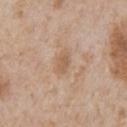| key | value |
|---|---|
| biopsy status | total-body-photography surveillance lesion; no biopsy |
| subject | male, in their mid-60s |
| diameter | ≈2.5 mm |
| site | the front of the torso |
| acquisition | total-body-photography crop, ~15 mm field of view |
| illumination | white-light |